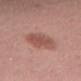Part of a total-body skin-imaging series; this lesion was reviewed on a skin check and was not flagged for biopsy. The tile uses white-light illumination. Cropped from a total-body skin-imaging series; the visible field is about 15 mm. A female patient about 40 years old. The lesion is on the leg.